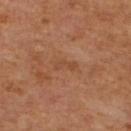biopsy_status: not biopsied; imaged during a skin examination